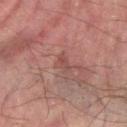A 15 mm close-up tile from a total-body photography series done for melanoma screening. A male patient aged around 75. The lesion is located on the right forearm.